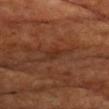Q: Was this lesion biopsied?
A: imaged on a skin check; not biopsied
Q: What is the anatomic site?
A: the chest
Q: Illumination type?
A: cross-polarized
Q: What kind of image is this?
A: 15 mm crop, total-body photography
Q: What did automated image analysis measure?
A: a footprint of about 3.5 mm², a shape eccentricity near 0.8, and a shape-asymmetry score of about 0.25 (0 = symmetric); an average lesion color of about L≈30 a*≈22 b*≈28 (CIELAB) and a normalized lesion–skin contrast near 5.5; radial color variation of about 1
Q: Patient demographics?
A: male, approximately 70 years of age
Q: Lesion size?
A: about 2.5 mm